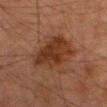biopsy status=catalogued during a skin exam; not biopsied | body site=the right thigh | illumination=cross-polarized | patient=male, aged approximately 80 | diameter=~6 mm (longest diameter) | image=15 mm crop, total-body photography.